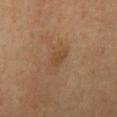Clinical impression: Imaged during a routine full-body skin examination; the lesion was not biopsied and no histopathology is available. Background: A male patient, in their 60s. Longest diameter approximately 2.5 mm. The tile uses cross-polarized illumination. The total-body-photography lesion software estimated a lesion color around L≈40 a*≈16 b*≈31 in CIELAB, roughly 6 lightness units darker than nearby skin, and a lesion-to-skin contrast of about 5.5 (normalized; higher = more distinct). And it measured a border-irregularity index near 2.5/10 and internal color variation of about 1 on a 0–10 scale. The software also gave a nevus-likeness score of about 0/100 and a detector confidence of about 100 out of 100 that the crop contains a lesion. The lesion is on the chest. Cropped from a total-body skin-imaging series; the visible field is about 15 mm.